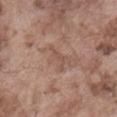This lesion was catalogued during total-body skin photography and was not selected for biopsy.
Located on the abdomen.
The patient is a male aged approximately 75.
Cropped from a whole-body photographic skin survey; the tile spans about 15 mm.